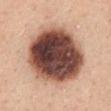• follow-up · no biopsy performed (imaged during a skin exam)
• image · ~15 mm crop, total-body skin-cancer survey
• patient · female, in their mid- to late 40s
• anatomic site · the mid back